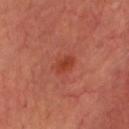Q: Is there a histopathology result?
A: imaged on a skin check; not biopsied
Q: Automated lesion metrics?
A: an area of roughly 4 mm², an outline eccentricity of about 0.65 (0 = round, 1 = elongated), and a symmetry-axis asymmetry near 0.2; roughly 7 lightness units darker than nearby skin and a normalized border contrast of about 6.5; a nevus-likeness score of about 85/100 and a detector confidence of about 100 out of 100 that the crop contains a lesion
Q: Lesion location?
A: the head or neck
Q: What are the patient's age and sex?
A: male, aged around 65
Q: What lighting was used for the tile?
A: cross-polarized illumination
Q: How large is the lesion?
A: about 2.5 mm
Q: What is the imaging modality?
A: ~15 mm tile from a whole-body skin photo